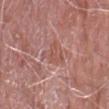follow-up: imaged on a skin check; not biopsied
diameter: ~2.5 mm (longest diameter)
illumination: white-light
automated lesion analysis: a lesion area of about 3.5 mm², a shape eccentricity near 0.6, and two-axis asymmetry of about 0.6; a mean CIELAB color near L≈53 a*≈24 b*≈26, a lesion–skin lightness drop of about 6, and a lesion-to-skin contrast of about 5 (normalized; higher = more distinct); a classifier nevus-likeness of about 0/100 and a lesion-detection confidence of about 95/100
patient: male, aged approximately 75
location: the right forearm
imaging modality: total-body-photography crop, ~15 mm field of view